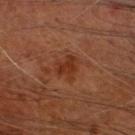notes: no biopsy performed (imaged during a skin exam) | lesion diameter: about 3 mm | patient: male, about 65 years old | automated lesion analysis: a lesion area of about 5 mm², an outline eccentricity of about 0.55 (0 = round, 1 = elongated), and a symmetry-axis asymmetry near 0.45; a border-irregularity rating of about 5/10, a within-lesion color-variation index near 2/10, and a peripheral color-asymmetry measure near 1; a classifier nevus-likeness of about 15/100 and a detector confidence of about 100 out of 100 that the crop contains a lesion | illumination: cross-polarized illumination | body site: the head or neck | acquisition: ~15 mm tile from a whole-body skin photo.